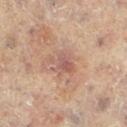Image and clinical context: About 3.5 mm across. A close-up tile cropped from a whole-body skin photograph, about 15 mm across. A female patient aged 78 to 82. The lesion is located on the right leg. This is a cross-polarized tile.